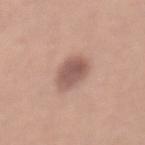biopsy status: catalogued during a skin exam; not biopsied
size: ~4 mm (longest diameter)
lighting: white-light illumination
subject: female, about 50 years old
body site: the abdomen
automated lesion analysis: a border-irregularity index near 1.5/10 and internal color variation of about 3 on a 0–10 scale
imaging modality: 15 mm crop, total-body photography